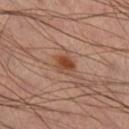| feature | finding |
|---|---|
| workup | total-body-photography surveillance lesion; no biopsy |
| illumination | cross-polarized illumination |
| subject | male, approximately 45 years of age |
| image source | total-body-photography crop, ~15 mm field of view |
| automated lesion analysis | an area of roughly 5 mm², a shape eccentricity near 0.55, and a shape-asymmetry score of about 0.2 (0 = symmetric); an average lesion color of about L≈42 a*≈21 b*≈30 (CIELAB), about 9 CIELAB-L* units darker than the surrounding skin, and a normalized lesion–skin contrast near 8.5; a border-irregularity rating of about 2/10, a color-variation rating of about 4.5/10, and a peripheral color-asymmetry measure near 1.5; a nevus-likeness score of about 95/100 |
| location | the right lower leg |